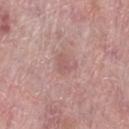The lesion was photographed on a routine skin check and not biopsied; there is no pathology result. Captured under white-light illumination. The total-body-photography lesion software estimated a mean CIELAB color near L≈57 a*≈22 b*≈21, a lesion–skin lightness drop of about 7, and a normalized border contrast of about 4.5. The analysis additionally found border irregularity of about 3.5 on a 0–10 scale, internal color variation of about 2 on a 0–10 scale, and peripheral color asymmetry of about 0.5. The analysis additionally found a nevus-likeness score of about 0/100 and a detector confidence of about 100 out of 100 that the crop contains a lesion. The recorded lesion diameter is about 2.5 mm. From the right lower leg. This image is a 15 mm lesion crop taken from a total-body photograph. A female patient approximately 60 years of age.